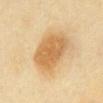• imaging modality — ~15 mm tile from a whole-body skin photo
• lesion size — about 6 mm
• subject — female, about 60 years old
• body site — the chest